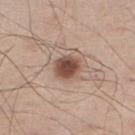{
  "patient": {
    "sex": "male",
    "age_approx": 60
  },
  "image": {
    "source": "total-body photography crop",
    "field_of_view_mm": 15
  },
  "lighting": "white-light",
  "site": "leg",
  "automated_metrics": {
    "cielab_L": 48,
    "cielab_a": 19,
    "cielab_b": 26,
    "vs_skin_darker_L": 16.0,
    "vs_skin_contrast_norm": 11.0,
    "border_irregularity_0_10": 1.5,
    "color_variation_0_10": 4.0,
    "peripheral_color_asymmetry": 1.0,
    "nevus_likeness_0_100": 100,
    "lesion_detection_confidence_0_100": 100
  },
  "lesion_size": {
    "long_diameter_mm_approx": 3.0
  }
}